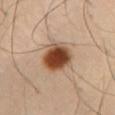Clinical impression: The lesion was photographed on a routine skin check and not biopsied; there is no pathology result. Context: The tile uses cross-polarized illumination. Longest diameter approximately 4 mm. The patient is a male roughly 55 years of age. On the abdomen. An algorithmic analysis of the crop reported an average lesion color of about L≈43 a*≈21 b*≈32 (CIELAB), roughly 19 lightness units darker than nearby skin, and a normalized border contrast of about 14. And it measured a border-irregularity rating of about 1.5/10, a within-lesion color-variation index near 7/10, and radial color variation of about 2. The software also gave a classifier nevus-likeness of about 100/100. A region of skin cropped from a whole-body photographic capture, roughly 15 mm wide.